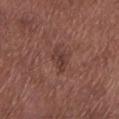No biopsy was performed on this lesion — it was imaged during a full skin examination and was not determined to be concerning.
An algorithmic analysis of the crop reported a footprint of about 4.5 mm² and a symmetry-axis asymmetry near 0.35. It also reported a mean CIELAB color near L≈38 a*≈20 b*≈23. The software also gave a nevus-likeness score of about 0/100 and a detector confidence of about 100 out of 100 that the crop contains a lesion.
A 15 mm close-up extracted from a 3D total-body photography capture.
A male patient aged 53–57.
On the left lower leg.
Approximately 3 mm at its widest.